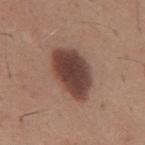Assessment:
This lesion was catalogued during total-body skin photography and was not selected for biopsy.
Acquisition and patient details:
A region of skin cropped from a whole-body photographic capture, roughly 15 mm wide. Captured under white-light illumination. On the back. A male subject in their mid- to late 60s.